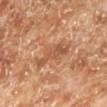Imaged during a routine full-body skin examination; the lesion was not biopsied and no histopathology is available. Captured under cross-polarized illumination. A region of skin cropped from a whole-body photographic capture, roughly 15 mm wide. The lesion is on the left lower leg. A male patient aged 68–72.